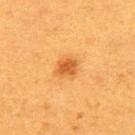Q: Was a biopsy performed?
A: catalogued during a skin exam; not biopsied
Q: What did automated image analysis measure?
A: a footprint of about 4 mm², a shape eccentricity near 0.7, and two-axis asymmetry of about 0.2; a lesion color around L≈48 a*≈25 b*≈42 in CIELAB, about 11 CIELAB-L* units darker than the surrounding skin, and a normalized border contrast of about 8; a classifier nevus-likeness of about 100/100 and a lesion-detection confidence of about 100/100
Q: What lighting was used for the tile?
A: cross-polarized illumination
Q: What are the patient's age and sex?
A: female, aged approximately 40
Q: What is the anatomic site?
A: the upper back
Q: How was this image acquired?
A: ~15 mm crop, total-body skin-cancer survey
Q: How large is the lesion?
A: about 2.5 mm